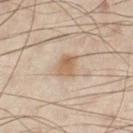Clinical impression:
The lesion was tiled from a total-body skin photograph and was not biopsied.
Background:
A male patient, aged approximately 50. The total-body-photography lesion software estimated an outline eccentricity of about 0.65 (0 = round, 1 = elongated) and a shape-asymmetry score of about 0.2 (0 = symmetric). It also reported a lesion color around L≈49 a*≈13 b*≈26 in CIELAB, a lesion–skin lightness drop of about 8, and a normalized border contrast of about 7. The analysis additionally found a border-irregularity index near 2/10. And it measured an automated nevus-likeness rating near 85 out of 100 and a lesion-detection confidence of about 100/100. A 15 mm close-up tile from a total-body photography series done for melanoma screening. The tile uses cross-polarized illumination. Located on the left lower leg.